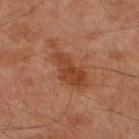Impression: This lesion was catalogued during total-body skin photography and was not selected for biopsy. Context: The recorded lesion diameter is about 7 mm. A male patient, aged 68 to 72. Cropped from a total-body skin-imaging series; the visible field is about 15 mm. Captured under cross-polarized illumination. Located on the right lower leg.